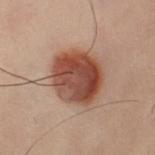  biopsy_status: not biopsied; imaged during a skin examination
  image:
    source: total-body photography crop
    field_of_view_mm: 15
  automated_metrics:
    cielab_L: 41
    cielab_a: 20
    cielab_b: 25
    vs_skin_darker_L: 13.0
    vs_skin_contrast_norm: 10.5
    border_irregularity_0_10: 2.5
    color_variation_0_10: 6.5
    peripheral_color_asymmetry: 2.0
  site: leg
  lighting: cross-polarized
  patient:
    sex: female
    age_approx: 60